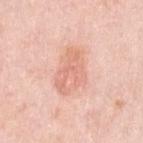Impression:
No biopsy was performed on this lesion — it was imaged during a full skin examination and was not determined to be concerning.
Acquisition and patient details:
From the left upper arm. A female patient roughly 65 years of age. A lesion tile, about 15 mm wide, cut from a 3D total-body photograph.